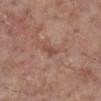follow-up = catalogued during a skin exam; not biopsied | image = ~15 mm tile from a whole-body skin photo | automated metrics = an average lesion color of about L≈48 a*≈23 b*≈27 (CIELAB) and about 8 CIELAB-L* units darker than the surrounding skin; a border-irregularity rating of about 4/10, internal color variation of about 0 on a 0–10 scale, and radial color variation of about 0; a classifier nevus-likeness of about 0/100 | anatomic site = the right lower leg | lighting = white-light | lesion size = about 2.5 mm | patient = female, aged approximately 75.